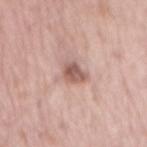Notes:
* notes · catalogued during a skin exam; not biopsied
* imaging modality · ~15 mm crop, total-body skin-cancer survey
* size · about 2.5 mm
* automated lesion analysis · a lesion area of about 4.5 mm² and two-axis asymmetry of about 0.3; a border-irregularity rating of about 2.5/10 and a peripheral color-asymmetry measure near 1.5
* subject · male, aged 63–67
* lighting · white-light
* body site · the lower back